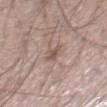This lesion was catalogued during total-body skin photography and was not selected for biopsy. A 15 mm close-up tile from a total-body photography series done for melanoma screening. The tile uses white-light illumination. From the right thigh. Longest diameter approximately 3 mm. Automated image analysis of the tile measured an average lesion color of about L≈54 a*≈16 b*≈22 (CIELAB), about 9 CIELAB-L* units darker than the surrounding skin, and a normalized lesion–skin contrast near 6.5. A male subject, approximately 55 years of age.